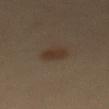biopsy status = catalogued during a skin exam; not biopsied
lesion size = ~3 mm (longest diameter)
illumination = cross-polarized
acquisition = total-body-photography crop, ~15 mm field of view
body site = the mid back
automated metrics = a mean CIELAB color near L≈33 a*≈14 b*≈24 and a normalized lesion–skin contrast near 7.5
subject = male, aged around 40